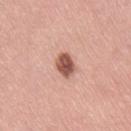{
  "site": "right thigh",
  "automated_metrics": {
    "color_variation_0_10": 3.0,
    "peripheral_color_asymmetry": 1.0,
    "lesion_detection_confidence_0_100": 100
  },
  "lighting": "white-light",
  "lesion_size": {
    "long_diameter_mm_approx": 3.5
  },
  "patient": {
    "sex": "female",
    "age_approx": 40
  },
  "image": {
    "source": "total-body photography crop",
    "field_of_view_mm": 15
  }
}